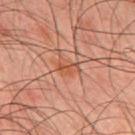notes: catalogued during a skin exam; not biopsied
subject: male, aged approximately 45
body site: the mid back
illumination: cross-polarized illumination
automated metrics: a lesion area of about 4 mm², an outline eccentricity of about 0.8 (0 = round, 1 = elongated), and two-axis asymmetry of about 0.3; a border-irregularity rating of about 2.5/10; a nevus-likeness score of about 5/100 and lesion-presence confidence of about 100/100
diameter: ~2.5 mm (longest diameter)
imaging modality: ~15 mm tile from a whole-body skin photo